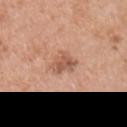Impression:
The lesion was tiled from a total-body skin photograph and was not biopsied.
Context:
The total-body-photography lesion software estimated an eccentricity of roughly 0.65 and a shape-asymmetry score of about 0.3 (0 = symmetric). The patient is a male aged 68 to 72. From the right upper arm. A close-up tile cropped from a whole-body skin photograph, about 15 mm across. Captured under white-light illumination. Longest diameter approximately 3 mm.